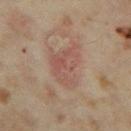| field | value |
|---|---|
| notes | total-body-photography surveillance lesion; no biopsy |
| lighting | cross-polarized illumination |
| body site | the left thigh |
| imaging modality | ~15 mm crop, total-body skin-cancer survey |
| diameter | about 5.5 mm |
| subject | female, approximately 35 years of age |